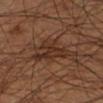{"biopsy_status": "not biopsied; imaged during a skin examination", "patient": {"sex": "male", "age_approx": 55}, "site": "left lower leg", "lesion_size": {"long_diameter_mm_approx": 6.5}, "image": {"source": "total-body photography crop", "field_of_view_mm": 15}}